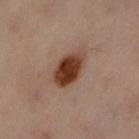Clinical impression: Captured during whole-body skin photography for melanoma surveillance; the lesion was not biopsied. Image and clinical context: The lesion is on the right leg. A roughly 15 mm field-of-view crop from a total-body skin photograph. The recorded lesion diameter is about 5 mm. A female subject aged 58–62. The tile uses cross-polarized illumination.